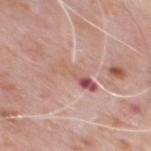Recorded during total-body skin imaging; not selected for excision or biopsy. From the chest. The patient is a male approximately 80 years of age. A 15 mm close-up extracted from a 3D total-body photography capture.